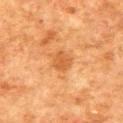notes: imaged on a skin check; not biopsied | image source: total-body-photography crop, ~15 mm field of view | lesion diameter: about 2.5 mm | patient: male, approximately 80 years of age | body site: the back | lighting: cross-polarized.